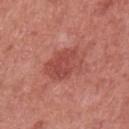Clinical impression:
Captured during whole-body skin photography for melanoma surveillance; the lesion was not biopsied.
Acquisition and patient details:
The lesion-visualizer software estimated a lesion area of about 14 mm² and an outline eccentricity of about 0.6 (0 = round, 1 = elongated). The software also gave a lesion-detection confidence of about 100/100. A female subject aged around 50. Measured at roughly 5 mm in maximum diameter. A close-up tile cropped from a whole-body skin photograph, about 15 mm across. Located on the left upper arm. Imaged with white-light lighting.